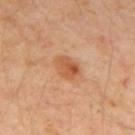Notes:
– biopsy status — total-body-photography surveillance lesion; no biopsy
– acquisition — total-body-photography crop, ~15 mm field of view
– anatomic site — the mid back
– lesion size — about 3 mm
– patient — male, about 55 years old